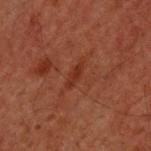Imaged during a routine full-body skin examination; the lesion was not biopsied and no histopathology is available. The lesion is located on the upper back. A male patient aged 58 to 62. About 2.5 mm across. A roughly 15 mm field-of-view crop from a total-body skin photograph. This is a cross-polarized tile. The total-body-photography lesion software estimated an outline eccentricity of about 0.95 (0 = round, 1 = elongated) and a shape-asymmetry score of about 0.25 (0 = symmetric).